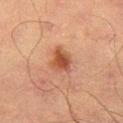Case summary:
• workup — total-body-photography surveillance lesion; no biopsy
• image source — ~15 mm tile from a whole-body skin photo
• patient — male, aged around 65
• automated metrics — a lesion area of about 6 mm² and an outline eccentricity of about 0.55 (0 = round, 1 = elongated); a lesion color around L≈41 a*≈22 b*≈30 in CIELAB, about 10 CIELAB-L* units darker than the surrounding skin, and a lesion-to-skin contrast of about 9 (normalized; higher = more distinct); peripheral color asymmetry of about 1
• body site — the right thigh